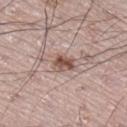Imaged during a routine full-body skin examination; the lesion was not biopsied and no histopathology is available.